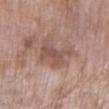| field | value |
|---|---|
| workup | no biopsy performed (imaged during a skin exam) |
| tile lighting | white-light |
| subject | female, aged 73–77 |
| image | ~15 mm crop, total-body skin-cancer survey |
| TBP lesion metrics | an area of roughly 7.5 mm², an outline eccentricity of about 0.8 (0 = round, 1 = elongated), and a shape-asymmetry score of about 0.35 (0 = symmetric); an automated nevus-likeness rating near 0 out of 100 and a detector confidence of about 100 out of 100 that the crop contains a lesion |
| lesion size | ~4 mm (longest diameter) |
| site | the left lower leg |